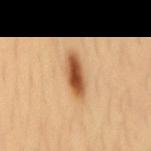Case summary:
• location: the mid back
• image: ~15 mm tile from a whole-body skin photo
• automated lesion analysis: a lesion area of about 8.5 mm², a shape eccentricity near 0.9, and a shape-asymmetry score of about 0.1 (0 = symmetric); an average lesion color of about L≈56 a*≈23 b*≈39 (CIELAB), roughly 17 lightness units darker than nearby skin, and a normalized border contrast of about 10.5
• subject: female, aged 33 to 37
• tile lighting: cross-polarized illumination
• lesion diameter: ~5 mm (longest diameter)